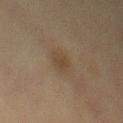The lesion was photographed on a routine skin check and not biopsied; there is no pathology result. An algorithmic analysis of the crop reported a shape eccentricity near 0.55 and a shape-asymmetry score of about 0.25 (0 = symmetric). The analysis additionally found a lesion–skin lightness drop of about 5. Imaged with cross-polarized lighting. A roughly 15 mm field-of-view crop from a total-body skin photograph. On the front of the torso. The patient is a female aged around 40.